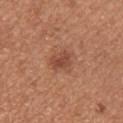The lesion was tiled from a total-body skin photograph and was not biopsied.
The total-body-photography lesion software estimated a footprint of about 4.5 mm², an outline eccentricity of about 0.7 (0 = round, 1 = elongated), and two-axis asymmetry of about 0.3. The software also gave about 9 CIELAB-L* units darker than the surrounding skin and a lesion-to-skin contrast of about 7 (normalized; higher = more distinct).
A 15 mm close-up extracted from a 3D total-body photography capture.
Measured at roughly 2.5 mm in maximum diameter.
From the arm.
A female patient approximately 25 years of age.
The tile uses white-light illumination.